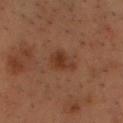Impression:
Imaged during a routine full-body skin examination; the lesion was not biopsied and no histopathology is available.
Clinical summary:
Cropped from a whole-body photographic skin survey; the tile spans about 15 mm. Automated tile analysis of the lesion measured a footprint of about 5.5 mm², an eccentricity of roughly 0.8, and a shape-asymmetry score of about 0.35 (0 = symmetric). It also reported an average lesion color of about L≈28 a*≈18 b*≈25 (CIELAB) and roughly 7 lightness units darker than nearby skin. The software also gave a border-irregularity index near 3.5/10, a color-variation rating of about 2/10, and a peripheral color-asymmetry measure near 0.5. The lesion is on the chest. About 3.5 mm across. A male subject, in their mid-50s.